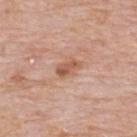<lesion>
<patient>
  <sex>male</sex>
  <age_approx>75</age_approx>
</patient>
<site>upper back</site>
<image>
  <source>total-body photography crop</source>
  <field_of_view_mm>15</field_of_view_mm>
</image>
<automated_metrics>
  <vs_skin_darker_L>10.0</vs_skin_darker_L>
  <vs_skin_contrast_norm>7.0</vs_skin_contrast_norm>
</automated_metrics>
<lighting>white-light</lighting>
<lesion_size>
  <long_diameter_mm_approx>3.0</long_diameter_mm_approx>
</lesion_size>
</lesion>